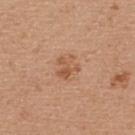{
  "biopsy_status": "not biopsied; imaged during a skin examination",
  "patient": {
    "sex": "male",
    "age_approx": 40
  },
  "site": "upper back",
  "image": {
    "source": "total-body photography crop",
    "field_of_view_mm": 15
  }
}